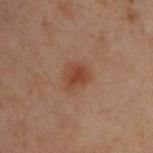Part of a total-body skin-imaging series; this lesion was reviewed on a skin check and was not flagged for biopsy.
A male patient aged 28–32.
A lesion tile, about 15 mm wide, cut from a 3D total-body photograph.
Approximately 3 mm at its widest.
The lesion-visualizer software estimated an average lesion color of about L≈35 a*≈19 b*≈25 (CIELAB), roughly 7 lightness units darker than nearby skin, and a normalized border contrast of about 7. And it measured an automated nevus-likeness rating near 90 out of 100.
The lesion is located on the upper back.
This is a cross-polarized tile.